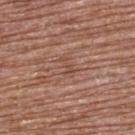Captured during whole-body skin photography for melanoma surveillance; the lesion was not biopsied.
An algorithmic analysis of the crop reported a lesion–skin lightness drop of about 6 and a lesion-to-skin contrast of about 5 (normalized; higher = more distinct). It also reported a border-irregularity index near 5/10, internal color variation of about 0 on a 0–10 scale, and radial color variation of about 0. It also reported a classifier nevus-likeness of about 0/100 and a lesion-detection confidence of about 70/100.
On the upper back.
A region of skin cropped from a whole-body photographic capture, roughly 15 mm wide.
Captured under white-light illumination.
The subject is a female aged 78–82.
The lesion's longest dimension is about 2.5 mm.